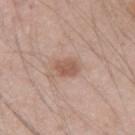The lesion was tiled from a total-body skin photograph and was not biopsied. The subject is a male in their mid-40s. Located on the left upper arm. A 15 mm close-up tile from a total-body photography series done for melanoma screening.